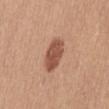This lesion was catalogued during total-body skin photography and was not selected for biopsy. Approximately 4 mm at its widest. Captured under white-light illumination. A 15 mm close-up tile from a total-body photography series done for melanoma screening. An algorithmic analysis of the crop reported a border-irregularity rating of about 2/10 and a peripheral color-asymmetry measure near 1. The patient is a female about 55 years old. On the abdomen.